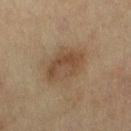The lesion was photographed on a routine skin check and not biopsied; there is no pathology result. A female patient, in their mid- to late 50s. The lesion's longest dimension is about 4.5 mm. Located on the right thigh. A roughly 15 mm field-of-view crop from a total-body skin photograph.